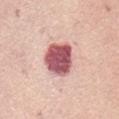follow-up: catalogued during a skin exam; not biopsied | image source: 15 mm crop, total-body photography | site: the abdomen | patient: female, about 55 years old | tile lighting: white-light.